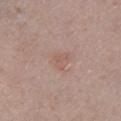| field | value |
|---|---|
| biopsy status | catalogued during a skin exam; not biopsied |
| anatomic site | the left lower leg |
| subject | female, aged 48 to 52 |
| lesion size | ≈4.5 mm |
| illumination | white-light illumination |
| image source | total-body-photography crop, ~15 mm field of view |